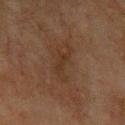follow-up = imaged on a skin check; not biopsied
acquisition = 15 mm crop, total-body photography
patient = male, roughly 75 years of age
size = ~3 mm (longest diameter)
lighting = cross-polarized
site = the left upper arm